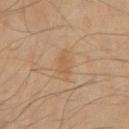Assessment: Imaged during a routine full-body skin examination; the lesion was not biopsied and no histopathology is available. Background: A close-up tile cropped from a whole-body skin photograph, about 15 mm across. A male patient, aged approximately 60. Captured under cross-polarized illumination. The lesion's longest dimension is about 3 mm. Located on the chest.